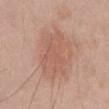Impression:
Part of a total-body skin-imaging series; this lesion was reviewed on a skin check and was not flagged for biopsy.
Background:
A male subject in their 50s. A roughly 15 mm field-of-view crop from a total-body skin photograph. Longest diameter approximately 5 mm. On the front of the torso.